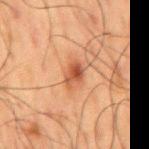Q: Where on the body is the lesion?
A: the mid back
Q: How large is the lesion?
A: ~3 mm (longest diameter)
Q: Automated lesion metrics?
A: an area of roughly 4.5 mm², a shape eccentricity near 0.8, and a shape-asymmetry score of about 0.35 (0 = symmetric); a border-irregularity index near 3.5/10, a within-lesion color-variation index near 4.5/10, and radial color variation of about 1.5
Q: Patient demographics?
A: male, aged 58 to 62
Q: How was this image acquired?
A: 15 mm crop, total-body photography